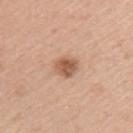workup = total-body-photography surveillance lesion; no biopsy | automated metrics = a lesion–skin lightness drop of about 13; a nevus-likeness score of about 90/100 and lesion-presence confidence of about 100/100 | anatomic site = the upper back | image = 15 mm crop, total-body photography | subject = male, aged around 35 | illumination = white-light illumination | lesion size = ≈3 mm.